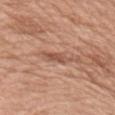Imaged during a routine full-body skin examination; the lesion was not biopsied and no histopathology is available. The lesion-visualizer software estimated a lesion-to-skin contrast of about 6.5 (normalized; higher = more distinct). It also reported a detector confidence of about 80 out of 100 that the crop contains a lesion. A 15 mm close-up tile from a total-body photography series done for melanoma screening. The lesion is on the right upper arm. The patient is a female aged approximately 55.